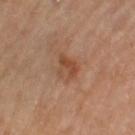The lesion was photographed on a routine skin check and not biopsied; there is no pathology result. The patient is a female aged 68–72. Measured at roughly 3 mm in maximum diameter. Cropped from a total-body skin-imaging series; the visible field is about 15 mm. The lesion is located on the left thigh. This is a cross-polarized tile.